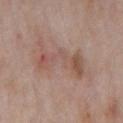Part of a total-body skin-imaging series; this lesion was reviewed on a skin check and was not flagged for biopsy.